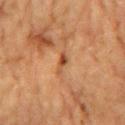Recorded during total-body skin imaging; not selected for excision or biopsy. A region of skin cropped from a whole-body photographic capture, roughly 15 mm wide. On the chest. Automated image analysis of the tile measured a footprint of about 2.5 mm², a shape eccentricity near 0.95, and two-axis asymmetry of about 0.4. The analysis additionally found about 10 CIELAB-L* units darker than the surrounding skin and a normalized lesion–skin contrast near 8. A male patient, about 85 years old or older. The recorded lesion diameter is about 3 mm. Imaged with cross-polarized lighting.